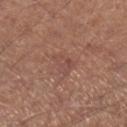The lesion was tiled from a total-body skin photograph and was not biopsied. Approximately 3 mm at its widest. A lesion tile, about 15 mm wide, cut from a 3D total-body photograph. Located on the right lower leg. Captured under white-light illumination. The subject is a male aged approximately 65.